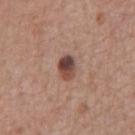This lesion was catalogued during total-body skin photography and was not selected for biopsy. Approximately 3 mm at its widest. A 15 mm close-up tile from a total-body photography series done for melanoma screening. The lesion is on the chest. The subject is a male approximately 65 years of age.